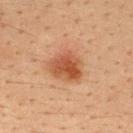No biopsy was performed on this lesion — it was imaged during a full skin examination and was not determined to be concerning. The lesion is on the back. A male patient, aged 33 to 37. A roughly 15 mm field-of-view crop from a total-body skin photograph. An algorithmic analysis of the crop reported a nevus-likeness score of about 100/100 and lesion-presence confidence of about 100/100. The lesion's longest dimension is about 4.5 mm.